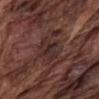Impression:
The lesion was photographed on a routine skin check and not biopsied; there is no pathology result.